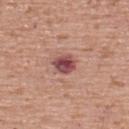Assessment: Part of a total-body skin-imaging series; this lesion was reviewed on a skin check and was not flagged for biopsy. Context: The lesion's longest dimension is about 2.5 mm. The patient is a male aged approximately 70. Imaged with white-light lighting. This image is a 15 mm lesion crop taken from a total-body photograph. Located on the upper back.